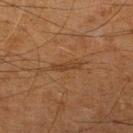notes: imaged on a skin check; not biopsied
subject: male, aged approximately 65
site: the leg
lesion size: ~3 mm (longest diameter)
image-analysis metrics: an area of roughly 2.5 mm² and two-axis asymmetry of about 0.4; a lesion color around L≈31 a*≈17 b*≈28 in CIELAB and about 6 CIELAB-L* units darker than the surrounding skin; a border-irregularity rating of about 5/10 and a peripheral color-asymmetry measure near 0; a nevus-likeness score of about 0/100
illumination: cross-polarized illumination
image: 15 mm crop, total-body photography